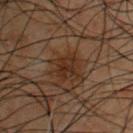Notes:
* follow-up — imaged on a skin check; not biopsied
* tile lighting — cross-polarized illumination
* anatomic site — the chest
* acquisition — ~15 mm crop, total-body skin-cancer survey
* subject — male, aged 48–52